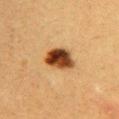Impression:
No biopsy was performed on this lesion — it was imaged during a full skin examination and was not determined to be concerning.
Acquisition and patient details:
A female patient, aged approximately 40. Captured under cross-polarized illumination. A 15 mm close-up extracted from a 3D total-body photography capture. From the right upper arm.